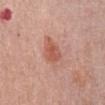biopsy_status: not biopsied; imaged during a skin examination
image:
  source: total-body photography crop
  field_of_view_mm: 15
site: chest
lesion_size:
  long_diameter_mm_approx: 4.5
patient:
  sex: female
  age_approx: 65